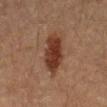Imaged during a routine full-body skin examination; the lesion was not biopsied and no histopathology is available.
Cropped from a total-body skin-imaging series; the visible field is about 15 mm.
A male patient, aged 58–62.
Imaged with cross-polarized lighting.
The total-body-photography lesion software estimated a footprint of about 12 mm², a shape eccentricity near 0.9, and two-axis asymmetry of about 0.2. It also reported a border-irregularity rating of about 3/10 and radial color variation of about 1. And it measured a nevus-likeness score of about 95/100 and a detector confidence of about 100 out of 100 that the crop contains a lesion.
The lesion is on the abdomen.